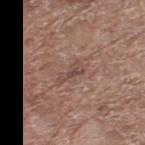Case summary:
- anatomic site: the left thigh
- patient: female, in their mid- to late 70s
- image source: 15 mm crop, total-body photography
- automated lesion analysis: an area of roughly 3.5 mm², a shape eccentricity near 0.9, and a shape-asymmetry score of about 0.45 (0 = symmetric); an average lesion color of about L≈46 a*≈18 b*≈22 (CIELAB), roughly 7 lightness units darker than nearby skin, and a lesion-to-skin contrast of about 6 (normalized; higher = more distinct)
- tile lighting: white-light